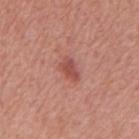Q: Was a biopsy performed?
A: total-body-photography surveillance lesion; no biopsy
Q: Who is the patient?
A: male, aged around 65
Q: What is the anatomic site?
A: the back
Q: How was this image acquired?
A: 15 mm crop, total-body photography